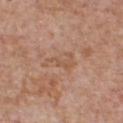Clinical impression: Captured during whole-body skin photography for melanoma surveillance; the lesion was not biopsied. Background: A male subject, about 65 years old. On the chest. The lesion-visualizer software estimated a mean CIELAB color near L≈55 a*≈20 b*≈31. Longest diameter approximately 4.5 mm. Cropped from a total-body skin-imaging series; the visible field is about 15 mm. The tile uses white-light illumination.